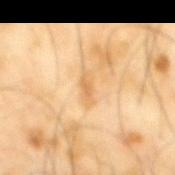Captured during whole-body skin photography for melanoma surveillance; the lesion was not biopsied. Cropped from a total-body skin-imaging series; the visible field is about 15 mm. Measured at roughly 3 mm in maximum diameter. Captured under cross-polarized illumination. The lesion is on the mid back. The patient is a male aged 63–67.